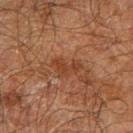Impression: Imaged during a routine full-body skin examination; the lesion was not biopsied and no histopathology is available. Context: This image is a 15 mm lesion crop taken from a total-body photograph. The total-body-photography lesion software estimated a lesion area of about 5 mm² and a shape-asymmetry score of about 0.45 (0 = symmetric). It also reported a mean CIELAB color near L≈33 a*≈21 b*≈28, a lesion–skin lightness drop of about 6, and a normalized lesion–skin contrast near 6.5. And it measured a border-irregularity index near 5.5/10 and peripheral color asymmetry of about 0.5. About 3.5 mm across. The lesion is located on the arm. A male subject, aged 68 to 72.